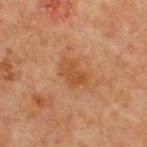Recorded during total-body skin imaging; not selected for excision or biopsy. Longest diameter approximately 4 mm. The subject is a male aged 63 to 67. A roughly 15 mm field-of-view crop from a total-body skin photograph. Imaged with cross-polarized lighting. The lesion is located on the front of the torso.